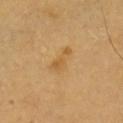<case>
  <biopsy_status>not biopsied; imaged during a skin examination</biopsy_status>
  <lesion_size>
    <long_diameter_mm_approx>3.5</long_diameter_mm_approx>
  </lesion_size>
  <image>
    <source>total-body photography crop</source>
    <field_of_view_mm>15</field_of_view_mm>
  </image>
  <lighting>cross-polarized</lighting>
  <automated_metrics>
    <area_mm2_approx>5.0</area_mm2_approx>
    <shape_asymmetry>0.4</shape_asymmetry>
    <color_variation_0_10>2.0</color_variation_0_10>
  </automated_metrics>
  <patient>
    <sex>male</sex>
    <age_approx>60</age_approx>
  </patient>
  <site>chest</site>
</case>